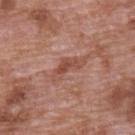Imaged during a routine full-body skin examination; the lesion was not biopsied and no histopathology is available. A 15 mm crop from a total-body photograph taken for skin-cancer surveillance. The lesion is on the back. A male subject aged 68 to 72.